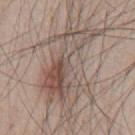The lesion was photographed on a routine skin check and not biopsied; there is no pathology result. The lesion is located on the chest. Automated tile analysis of the lesion measured a lesion area of about 37 mm², a shape eccentricity near 0.9, and a shape-asymmetry score of about 0.35 (0 = symmetric). The analysis additionally found a lesion color around L≈53 a*≈13 b*≈22 in CIELAB and a normalized lesion–skin contrast near 6.5. The software also gave internal color variation of about 10 on a 0–10 scale and a peripheral color-asymmetry measure near 3.5. The analysis additionally found an automated nevus-likeness rating near 5 out of 100 and a detector confidence of about 55 out of 100 that the crop contains a lesion. The recorded lesion diameter is about 11 mm. The patient is a male approximately 45 years of age. A 15 mm crop from a total-body photograph taken for skin-cancer surveillance.